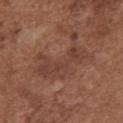  biopsy_status: not biopsied; imaged during a skin examination
  lesion_size:
    long_diameter_mm_approx: 7.0
  site: chest
  patient:
    sex: female
    age_approx: 75
  lighting: white-light
  image:
    source: total-body photography crop
    field_of_view_mm: 15
  automated_metrics:
    area_mm2_approx: 16.0
    eccentricity: 0.85
    shape_asymmetry: 0.55
    cielab_L: 41
    cielab_a: 22
    cielab_b: 26
    vs_skin_darker_L: 6.0
    vs_skin_contrast_norm: 5.5
    lesion_detection_confidence_0_100: 100